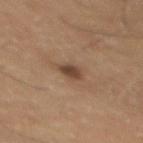No biopsy was performed on this lesion — it was imaged during a full skin examination and was not determined to be concerning.
Longest diameter approximately 2.5 mm.
A male patient approximately 60 years of age.
Imaged with cross-polarized lighting.
An algorithmic analysis of the crop reported an area of roughly 3 mm², an outline eccentricity of about 0.8 (0 = round, 1 = elongated), and a shape-asymmetry score of about 0.35 (0 = symmetric).
This image is a 15 mm lesion crop taken from a total-body photograph.